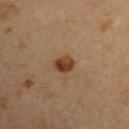Assessment: Imaged during a routine full-body skin examination; the lesion was not biopsied and no histopathology is available. Background: About 2.5 mm across. A lesion tile, about 15 mm wide, cut from a 3D total-body photograph. A male subject, about 55 years old. The lesion is located on the left upper arm.